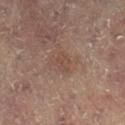notes=no biopsy performed (imaged during a skin exam) | patient=female, aged approximately 80 | illumination=cross-polarized illumination | acquisition=15 mm crop, total-body photography | body site=the left leg | automated lesion analysis=a border-irregularity index near 3/10 and internal color variation of about 2 on a 0–10 scale.